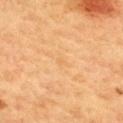biopsy status = catalogued during a skin exam; not biopsied | diameter = ~1.5 mm (longest diameter) | illumination = cross-polarized | subject = female, in their 60s | image = total-body-photography crop, ~15 mm field of view | site = the upper back | automated lesion analysis = an average lesion color of about L≈62 a*≈19 b*≈40 (CIELAB) and a lesion–skin lightness drop of about 3; a border-irregularity index near 3/10 and peripheral color asymmetry of about 0; a classifier nevus-likeness of about 0/100 and a detector confidence of about 100 out of 100 that the crop contains a lesion.